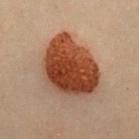{
  "biopsy_status": "not biopsied; imaged during a skin examination",
  "lighting": "cross-polarized",
  "site": "chest",
  "lesion_size": {
    "long_diameter_mm_approx": 7.5
  },
  "automated_metrics": {
    "vs_skin_darker_L": 15.0,
    "vs_skin_contrast_norm": 13.5,
    "nevus_likeness_0_100": 100,
    "lesion_detection_confidence_0_100": 100
  },
  "image": {
    "source": "total-body photography crop",
    "field_of_view_mm": 15
  },
  "patient": {
    "sex": "female",
    "age_approx": 40
  }
}